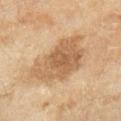workup = imaged on a skin check; not biopsied
TBP lesion metrics = an automated nevus-likeness rating near 5 out of 100 and a lesion-detection confidence of about 100/100
illumination = cross-polarized
site = the right lower leg
image source = total-body-photography crop, ~15 mm field of view
patient = female, in their 60s